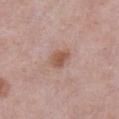The subject is a male aged 53–57.
On the abdomen.
Captured under white-light illumination.
Automated image analysis of the tile measured border irregularity of about 2 on a 0–10 scale, a color-variation rating of about 2/10, and radial color variation of about 0.5. And it measured an automated nevus-likeness rating near 75 out of 100.
The lesion's longest dimension is about 2.5 mm.
A 15 mm crop from a total-body photograph taken for skin-cancer surveillance.